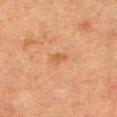{
  "biopsy_status": "not biopsied; imaged during a skin examination",
  "patient": {
    "sex": "female",
    "age_approx": 50
  },
  "image": {
    "source": "total-body photography crop",
    "field_of_view_mm": 15
  },
  "site": "abdomen",
  "lighting": "cross-polarized",
  "automated_metrics": {
    "area_mm2_approx": 3.0,
    "eccentricity": 0.85,
    "shape_asymmetry": 0.3,
    "cielab_L": 48,
    "cielab_a": 20,
    "cielab_b": 34,
    "vs_skin_darker_L": 6.0,
    "vs_skin_contrast_norm": 5.5,
    "color_variation_0_10": 1.0,
    "peripheral_color_asymmetry": 0.5
  },
  "lesion_size": {
    "long_diameter_mm_approx": 2.5
  }
}